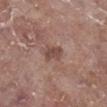Clinical impression:
This lesion was catalogued during total-body skin photography and was not selected for biopsy.
Clinical summary:
Captured under white-light illumination. A female patient, about 75 years old. From the right lower leg. A 15 mm close-up extracted from a 3D total-body photography capture. About 3 mm across. Automated image analysis of the tile measured an automated nevus-likeness rating near 0 out of 100 and a lesion-detection confidence of about 100/100.